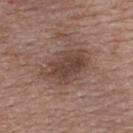Captured during whole-body skin photography for melanoma surveillance; the lesion was not biopsied. From the back. A 15 mm close-up tile from a total-body photography series done for melanoma screening. A female patient, aged 48 to 52. The tile uses white-light illumination. The lesion-visualizer software estimated a footprint of about 18 mm² and a symmetry-axis asymmetry near 0.25. The analysis additionally found a within-lesion color-variation index near 4/10 and a peripheral color-asymmetry measure near 1.5. It also reported a nevus-likeness score of about 55/100.